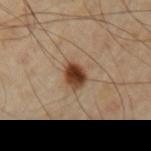Clinical impression:
The lesion was photographed on a routine skin check and not biopsied; there is no pathology result.
Background:
This image is a 15 mm lesion crop taken from a total-body photograph. An algorithmic analysis of the crop reported a lesion area of about 5.5 mm², an eccentricity of roughly 0.6, and two-axis asymmetry of about 0.25. The software also gave a detector confidence of about 100 out of 100 that the crop contains a lesion. The patient is a male about 65 years old. Captured under cross-polarized illumination. The lesion is located on the left upper arm.